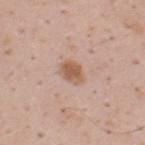Q: What is the imaging modality?
A: ~15 mm crop, total-body skin-cancer survey
Q: Who is the patient?
A: male, approximately 35 years of age
Q: Lesion location?
A: the upper back
Q: Automated lesion metrics?
A: an average lesion color of about L≈58 a*≈20 b*≈30 (CIELAB) and a lesion–skin lightness drop of about 11; lesion-presence confidence of about 100/100
Q: How was the tile lit?
A: white-light illumination